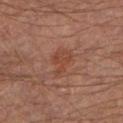| field | value |
|---|---|
| workup | imaged on a skin check; not biopsied |
| acquisition | ~15 mm tile from a whole-body skin photo |
| location | the left lower leg |
| patient | male, aged around 65 |